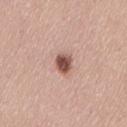workup=catalogued during a skin exam; not biopsied | site=the back | automated lesion analysis=a mean CIELAB color near L≈51 a*≈22 b*≈25, a lesion–skin lightness drop of about 17, and a normalized border contrast of about 11.5; a border-irregularity index near 1.5/10 and internal color variation of about 3.5 on a 0–10 scale | image=15 mm crop, total-body photography | lighting=white-light | subject=female, aged around 30 | diameter=≈2.5 mm.